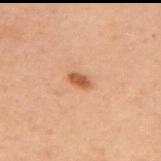notes: total-body-photography surveillance lesion; no biopsy
imaging modality: total-body-photography crop, ~15 mm field of view
site: the upper back
subject: female, about 55 years old